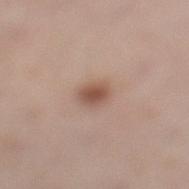Assessment:
Imaged during a routine full-body skin examination; the lesion was not biopsied and no histopathology is available.
Clinical summary:
A female patient roughly 40 years of age. This image is a 15 mm lesion crop taken from a total-body photograph. An algorithmic analysis of the crop reported a footprint of about 4.5 mm², a shape eccentricity near 0.7, and two-axis asymmetry of about 0.2. The analysis additionally found a color-variation rating of about 3/10 and peripheral color asymmetry of about 1. And it measured lesion-presence confidence of about 100/100. Captured under white-light illumination. Located on the left lower leg. Measured at roughly 3 mm in maximum diameter.